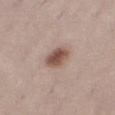{"biopsy_status": "not biopsied; imaged during a skin examination", "lighting": "white-light", "site": "right thigh", "lesion_size": {"long_diameter_mm_approx": 3.5}, "patient": {"sex": "female", "age_approx": 30}, "image": {"source": "total-body photography crop", "field_of_view_mm": 15}, "automated_metrics": {"cielab_L": 51, "cielab_a": 19, "cielab_b": 25, "vs_skin_darker_L": 14.0, "vs_skin_contrast_norm": 9.5, "nevus_likeness_0_100": 95, "lesion_detection_confidence_0_100": 100}}